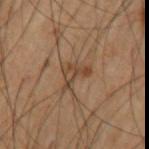Clinical impression: The lesion was photographed on a routine skin check and not biopsied; there is no pathology result. Context: A roughly 15 mm field-of-view crop from a total-body skin photograph. From the left upper arm. Captured under cross-polarized illumination. A male subject aged 58 to 62.